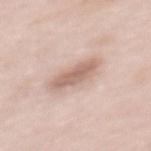- follow-up — imaged on a skin check; not biopsied
- patient — female, approximately 65 years of age
- size — ~5 mm (longest diameter)
- tile lighting — white-light illumination
- imaging modality — 15 mm crop, total-body photography
- location — the mid back
- TBP lesion metrics — an area of roughly 8.5 mm², an outline eccentricity of about 0.9 (0 = round, 1 = elongated), and a shape-asymmetry score of about 0.2 (0 = symmetric); a mean CIELAB color near L≈64 a*≈18 b*≈26 and about 12 CIELAB-L* units darker than the surrounding skin; a nevus-likeness score of about 25/100 and a lesion-detection confidence of about 95/100